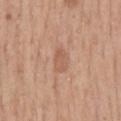Recorded during total-body skin imaging; not selected for excision or biopsy. On the back. A close-up tile cropped from a whole-body skin photograph, about 15 mm across. A male subject aged around 55.